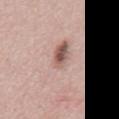follow-up: no biopsy performed (imaged during a skin exam); imaging modality: 15 mm crop, total-body photography; location: the chest; illumination: white-light illumination; lesion diameter: about 7.5 mm; subject: male, aged 48–52.